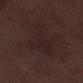Q: Is there a histopathology result?
A: imaged on a skin check; not biopsied
Q: What lighting was used for the tile?
A: white-light
Q: What are the patient's age and sex?
A: male, aged around 70
Q: What did automated image analysis measure?
A: an average lesion color of about L≈20 a*≈14 b*≈14 (CIELAB), about 3 CIELAB-L* units darker than the surrounding skin, and a normalized border contrast of about 4.5; an automated nevus-likeness rating near 0 out of 100 and a detector confidence of about 85 out of 100 that the crop contains a lesion
Q: What is the imaging modality?
A: total-body-photography crop, ~15 mm field of view
Q: Lesion location?
A: the leg
Q: Lesion size?
A: ~6.5 mm (longest diameter)